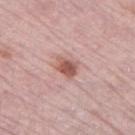Notes:
• notes — no biopsy performed (imaged during a skin exam)
• patient — female, in their mid- to late 60s
• body site — the left thigh
• acquisition — ~15 mm crop, total-body skin-cancer survey